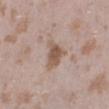{
  "biopsy_status": "not biopsied; imaged during a skin examination",
  "image": {
    "source": "total-body photography crop",
    "field_of_view_mm": 15
  },
  "automated_metrics": {
    "area_mm2_approx": 7.5,
    "eccentricity": 0.65,
    "shape_asymmetry": 0.4,
    "border_irregularity_0_10": 4.0,
    "color_variation_0_10": 3.0,
    "peripheral_color_asymmetry": 1.0
  },
  "site": "right lower leg",
  "lighting": "white-light",
  "patient": {
    "sex": "female",
    "age_approx": 25
  }
}